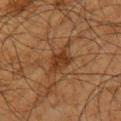| field | value |
|---|---|
| biopsy status | catalogued during a skin exam; not biopsied |
| anatomic site | the arm |
| patient | male, aged approximately 65 |
| lesion diameter | ~4 mm (longest diameter) |
| image source | ~15 mm crop, total-body skin-cancer survey |
| illumination | cross-polarized illumination |
| image-analysis metrics | a border-irregularity index near 4/10, a within-lesion color-variation index near 2.5/10, and radial color variation of about 1 |